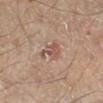workup: imaged on a skin check; not biopsied | patient: male, aged 63 to 67 | lesion diameter: ≈3 mm | acquisition: total-body-photography crop, ~15 mm field of view | location: the right lower leg.